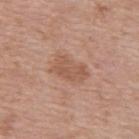biopsy status — total-body-photography surveillance lesion; no biopsy | tile lighting — white-light | diameter — about 4 mm | subject — male, aged approximately 55 | acquisition — ~15 mm tile from a whole-body skin photo | location — the back | TBP lesion metrics — an average lesion color of about L≈55 a*≈21 b*≈29 (CIELAB) and a normalized border contrast of about 5.5; a border-irregularity index near 2.5/10 and a within-lesion color-variation index near 2.5/10; a nevus-likeness score of about 0/100 and a detector confidence of about 100 out of 100 that the crop contains a lesion.